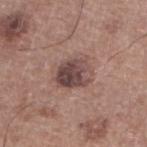follow-up: catalogued during a skin exam; not biopsied
image: total-body-photography crop, ~15 mm field of view
patient: male, aged around 65
location: the left lower leg
diameter: ~4 mm (longest diameter)